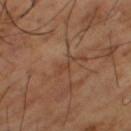Q: Was this lesion biopsied?
A: catalogued during a skin exam; not biopsied
Q: How was the tile lit?
A: cross-polarized
Q: What kind of image is this?
A: 15 mm crop, total-body photography
Q: Patient demographics?
A: male, aged 63–67
Q: How large is the lesion?
A: ≈2.5 mm
Q: What is the anatomic site?
A: the left thigh